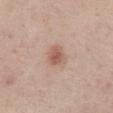Q: What are the patient's age and sex?
A: male, approximately 55 years of age
Q: What is the anatomic site?
A: the mid back
Q: Illumination type?
A: white-light
Q: What kind of image is this?
A: 15 mm crop, total-body photography
Q: How large is the lesion?
A: about 3 mm